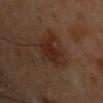Case summary:
• notes — no biopsy performed (imaged during a skin exam)
• patient — male, approximately 45 years of age
• acquisition — ~15 mm tile from a whole-body skin photo
• location — the arm
• lesion diameter — ≈5 mm